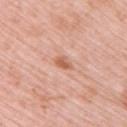patient:
  sex: female
  age_approx: 65
site: upper back
image:
  source: total-body photography crop
  field_of_view_mm: 15